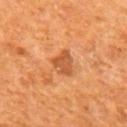• workup: no biopsy performed (imaged during a skin exam)
• subject: male, in their mid- to late 60s
• body site: the mid back
• imaging modality: total-body-photography crop, ~15 mm field of view